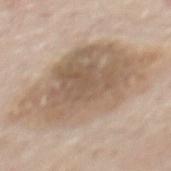| key | value |
|---|---|
| biopsy status | no biopsy performed (imaged during a skin exam) |
| automated lesion analysis | a mean CIELAB color near L≈60 a*≈13 b*≈28, about 11 CIELAB-L* units darker than the surrounding skin, and a normalized lesion–skin contrast near 7.5 |
| location | the mid back |
| image source | ~15 mm tile from a whole-body skin photo |
| subject | male, roughly 55 years of age |
| lighting | white-light |